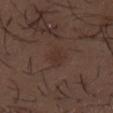Part of a total-body skin-imaging series; this lesion was reviewed on a skin check and was not flagged for biopsy.
A male patient approximately 50 years of age.
A 15 mm close-up tile from a total-body photography series done for melanoma screening.
About 3.5 mm across.
Located on the mid back.